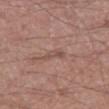{"biopsy_status": "not biopsied; imaged during a skin examination", "automated_metrics": {"area_mm2_approx": 3.0, "shape_asymmetry": 0.5, "cielab_L": 50, "cielab_a": 19, "cielab_b": 24, "vs_skin_darker_L": 7.0, "vs_skin_contrast_norm": 5.0, "nevus_likeness_0_100": 0, "lesion_detection_confidence_0_100": 90}, "site": "right thigh", "patient": {"sex": "male", "age_approx": 55}, "lighting": "white-light", "lesion_size": {"long_diameter_mm_approx": 2.5}, "image": {"source": "total-body photography crop", "field_of_view_mm": 15}}